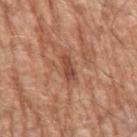notes — imaged on a skin check; not biopsied | anatomic site — the left upper arm | automated lesion analysis — an eccentricity of roughly 0.95; an average lesion color of about L≈47 a*≈24 b*≈30 (CIELAB), a lesion–skin lightness drop of about 11, and a normalized lesion–skin contrast near 8 | acquisition — ~15 mm tile from a whole-body skin photo | subject — male, aged approximately 65 | diameter — ~3 mm (longest diameter).